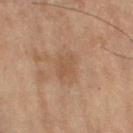Background: The lesion is located on the left thigh. Approximately 3 mm at its widest. Automated image analysis of the tile measured a lesion color around L≈39 a*≈15 b*≈25 in CIELAB, about 5 CIELAB-L* units darker than the surrounding skin, and a lesion-to-skin contrast of about 4.5 (normalized; higher = more distinct). The software also gave border irregularity of about 2.5 on a 0–10 scale and a peripheral color-asymmetry measure near 1. This is a cross-polarized tile. The patient is a male in their 70s. Cropped from a whole-body photographic skin survey; the tile spans about 15 mm.